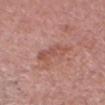notes: imaged on a skin check; not biopsied
patient: male, aged around 75
location: the head or neck
tile lighting: white-light illumination
acquisition: total-body-photography crop, ~15 mm field of view
diameter: about 4.5 mm